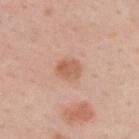workup = no biopsy performed (imaged during a skin exam) | tile lighting = white-light | image source = 15 mm crop, total-body photography | site = the upper back | lesion size = about 3 mm | subject = male, approximately 60 years of age.